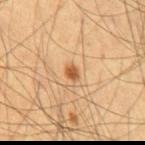Imaged with cross-polarized lighting.
Approximately 1.5 mm at its widest.
The patient is a male about 55 years old.
Located on the abdomen.
A 15 mm close-up extracted from a 3D total-body photography capture.